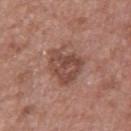workup=imaged on a skin check; not biopsied | acquisition=~15 mm crop, total-body skin-cancer survey | illumination=white-light illumination | patient=male, aged around 50 | site=the mid back | lesion size=~4.5 mm (longest diameter).